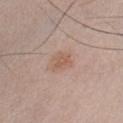Recorded during total-body skin imaging; not selected for excision or biopsy. A 15 mm close-up extracted from a 3D total-body photography capture. A male patient, in their mid-20s. Approximately 3 mm at its widest. Imaged with white-light lighting. The lesion-visualizer software estimated an eccentricity of roughly 0.65 and a shape-asymmetry score of about 0.3 (0 = symmetric). It also reported an average lesion color of about L≈57 a*≈19 b*≈28 (CIELAB), roughly 7 lightness units darker than nearby skin, and a normalized lesion–skin contrast near 6. The software also gave a classifier nevus-likeness of about 55/100 and a lesion-detection confidence of about 100/100. The lesion is located on the front of the torso.